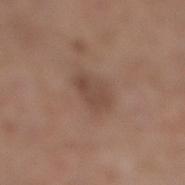Recorded during total-body skin imaging; not selected for excision or biopsy. The recorded lesion diameter is about 3.5 mm. Imaged with white-light lighting. Located on the leg. Automated image analysis of the tile measured an area of roughly 7.5 mm², an eccentricity of roughly 0.65, and a symmetry-axis asymmetry near 0.2. The software also gave a border-irregularity rating of about 2/10, internal color variation of about 2.5 on a 0–10 scale, and radial color variation of about 1. And it measured an automated nevus-likeness rating near 5 out of 100 and a detector confidence of about 100 out of 100 that the crop contains a lesion. A close-up tile cropped from a whole-body skin photograph, about 15 mm across. A female patient, about 65 years old.